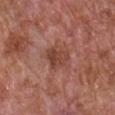Clinical impression:
This lesion was catalogued during total-body skin photography and was not selected for biopsy.
Acquisition and patient details:
From the chest. The tile uses white-light illumination. A region of skin cropped from a whole-body photographic capture, roughly 15 mm wide. Approximately 3 mm at its widest. A male subject about 65 years old.